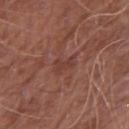Clinical impression:
Part of a total-body skin-imaging series; this lesion was reviewed on a skin check and was not flagged for biopsy.
Image and clinical context:
The subject is a male aged 63–67. A 15 mm close-up tile from a total-body photography series done for melanoma screening. The lesion is on the right upper arm.